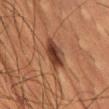Image and clinical context: Longest diameter approximately 5 mm. Cropped from a total-body skin-imaging series; the visible field is about 15 mm. The patient is a male aged around 55. The lesion-visualizer software estimated an area of roughly 7.5 mm², an eccentricity of roughly 0.9, and two-axis asymmetry of about 0.2. And it measured an average lesion color of about L≈34 a*≈20 b*≈27 (CIELAB), a lesion–skin lightness drop of about 12, and a lesion-to-skin contrast of about 10.5 (normalized; higher = more distinct). From the lower back. The tile uses cross-polarized illumination.